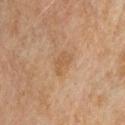Q: Is there a histopathology result?
A: total-body-photography surveillance lesion; no biopsy
Q: What did automated image analysis measure?
A: a shape eccentricity near 0.85 and two-axis asymmetry of about 0.4; a mean CIELAB color near L≈48 a*≈16 b*≈31, a lesion–skin lightness drop of about 6, and a normalized border contrast of about 5.5; a border-irregularity index near 4/10, internal color variation of about 1 on a 0–10 scale, and peripheral color asymmetry of about 0.5; a nevus-likeness score of about 0/100 and a lesion-detection confidence of about 100/100
Q: Lesion location?
A: the head or neck
Q: How was this image acquired?
A: total-body-photography crop, ~15 mm field of view
Q: Lesion size?
A: ~3.5 mm (longest diameter)
Q: What are the patient's age and sex?
A: female, roughly 70 years of age